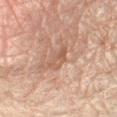Q: How was this image acquired?
A: ~15 mm tile from a whole-body skin photo
Q: How large is the lesion?
A: ~4 mm (longest diameter)
Q: Patient demographics?
A: male, in their mid- to late 40s
Q: Where on the body is the lesion?
A: the right forearm